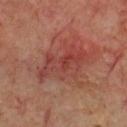Q: Was a biopsy performed?
A: total-body-photography surveillance lesion; no biopsy
Q: What did automated image analysis measure?
A: an average lesion color of about L≈41 a*≈28 b*≈26 (CIELAB), about 7 CIELAB-L* units darker than the surrounding skin, and a normalized lesion–skin contrast near 6
Q: What is the imaging modality?
A: ~15 mm crop, total-body skin-cancer survey
Q: How large is the lesion?
A: ≈6.5 mm
Q: Lesion location?
A: the chest
Q: Who is the patient?
A: male, about 70 years old
Q: Illumination type?
A: cross-polarized illumination